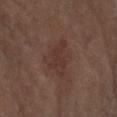Clinical impression:
Captured during whole-body skin photography for melanoma surveillance; the lesion was not biopsied.
Background:
A female subject aged 68 to 72. A lesion tile, about 15 mm wide, cut from a 3D total-body photograph. On the left forearm. The lesion's longest dimension is about 5 mm. An algorithmic analysis of the crop reported a lesion area of about 10 mm² and two-axis asymmetry of about 0.45.